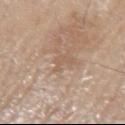biopsy_status: not biopsied; imaged during a skin examination
automated_metrics:
  cielab_L: 57
  cielab_a: 17
  cielab_b: 29
  vs_skin_darker_L: 6.0
  vs_skin_contrast_norm: 4.5
  nevus_likeness_0_100: 0
  lesion_detection_confidence_0_100: 100
site: arm
patient:
  sex: male
  age_approx: 80
lesion_size:
  long_diameter_mm_approx: 2.5
image:
  source: total-body photography crop
  field_of_view_mm: 15
lighting: white-light